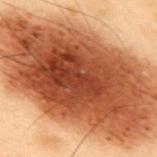Case summary:
• workup: total-body-photography surveillance lesion; no biopsy
• automated lesion analysis: an area of roughly 115 mm² and an eccentricity of roughly 0.85; a mean CIELAB color near L≈41 a*≈24 b*≈32, a lesion–skin lightness drop of about 20, and a normalized border contrast of about 14.5; a within-lesion color-variation index near 7.5/10 and peripheral color asymmetry of about 2.5
• body site: the back
• imaging modality: 15 mm crop, total-body photography
• diameter: ≈17.5 mm
• patient: male, aged 53–57
• lighting: cross-polarized